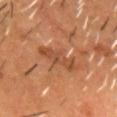| key | value |
|---|---|
| workup | catalogued during a skin exam; not biopsied |
| subject | male, aged approximately 55 |
| image-analysis metrics | a footprint of about 8 mm², an eccentricity of roughly 0.9, and a shape-asymmetry score of about 0.3 (0 = symmetric); a mean CIELAB color near L≈44 a*≈23 b*≈33, a lesion–skin lightness drop of about 8, and a lesion-to-skin contrast of about 6 (normalized; higher = more distinct); an automated nevus-likeness rating near 0 out of 100 |
| lighting | cross-polarized |
| image | ~15 mm crop, total-body skin-cancer survey |
| lesion diameter | ~4.5 mm (longest diameter) |
| site | the head or neck |